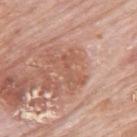Clinical impression:
Imaged during a routine full-body skin examination; the lesion was not biopsied and no histopathology is available.
Image and clinical context:
On the mid back. About 5 mm across. The total-body-photography lesion software estimated an average lesion color of about L≈57 a*≈23 b*≈30 (CIELAB), roughly 7 lightness units darker than nearby skin, and a normalized lesion–skin contrast near 5.5. It also reported a within-lesion color-variation index near 2.5/10 and a peripheral color-asymmetry measure near 1. A male patient, roughly 80 years of age. A 15 mm close-up extracted from a 3D total-body photography capture. This is a white-light tile.